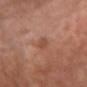Background: Imaged with white-light lighting. The lesion is on the chest. A female subject in their mid-70s. Cropped from a total-body skin-imaging series; the visible field is about 15 mm. About 2.5 mm across. The total-body-photography lesion software estimated a border-irregularity rating of about 3/10, a within-lesion color-variation index near 1.5/10, and radial color variation of about 0.5.